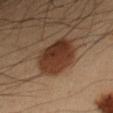Part of a total-body skin-imaging series; this lesion was reviewed on a skin check and was not flagged for biopsy. On the left forearm. A 15 mm close-up extracted from a 3D total-body photography capture. A male patient, aged 53 to 57.